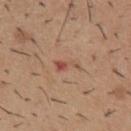notes: total-body-photography surveillance lesion; no biopsy | tile lighting: white-light illumination | size: ~3 mm (longest diameter) | image source: 15 mm crop, total-body photography | location: the mid back | image-analysis metrics: about 9 CIELAB-L* units darker than the surrounding skin and a normalized border contrast of about 6; a color-variation rating of about 1/10 and peripheral color asymmetry of about 0.5; a nevus-likeness score of about 0/100 and a detector confidence of about 100 out of 100 that the crop contains a lesion | subject: male, aged 38–42.